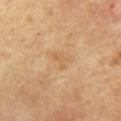Assessment:
Imaged during a routine full-body skin examination; the lesion was not biopsied and no histopathology is available.
Context:
The lesion is on the chest. Measured at roughly 2.5 mm in maximum diameter. A male subject, aged around 65. Cropped from a total-body skin-imaging series; the visible field is about 15 mm.